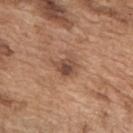{
  "biopsy_status": "not biopsied; imaged during a skin examination",
  "lighting": "white-light",
  "site": "upper back",
  "lesion_size": {
    "long_diameter_mm_approx": 3.0
  },
  "image": {
    "source": "total-body photography crop",
    "field_of_view_mm": 15
  },
  "patient": {
    "sex": "male",
    "age_approx": 75
  }
}